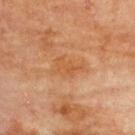Case summary:
* notes — total-body-photography surveillance lesion; no biopsy
* location — the upper back
* automated lesion analysis — a within-lesion color-variation index near 2/10 and a peripheral color-asymmetry measure near 1
* diameter — about 4 mm
* patient — female, in their 80s
* image source — ~15 mm crop, total-body skin-cancer survey
* illumination — cross-polarized